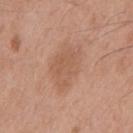workup: no biopsy performed (imaged during a skin exam).